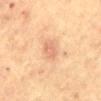• site — the abdomen
• subject — male, aged 63–67
• lesion size — ≈2.5 mm
• tile lighting — cross-polarized
• acquisition — total-body-photography crop, ~15 mm field of view